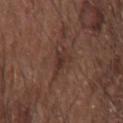A 15 mm close-up tile from a total-body photography series done for melanoma screening. Located on the left forearm. About 3.5 mm across. The tile uses white-light illumination. A male patient, aged approximately 65.